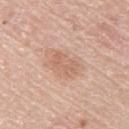* location · the upper back
* image · ~15 mm tile from a whole-body skin photo
* patient · male, aged 58 to 62